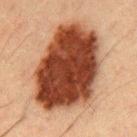Imaged during a routine full-body skin examination; the lesion was not biopsied and no histopathology is available.
A lesion tile, about 15 mm wide, cut from a 3D total-body photograph.
A male subject, aged 48 to 52.
From the mid back.